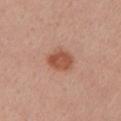biopsy status — total-body-photography surveillance lesion; no biopsy
lesion size — about 3.5 mm
acquisition — ~15 mm tile from a whole-body skin photo
lighting — white-light illumination
subject — female, aged 53–57
location — the left upper arm